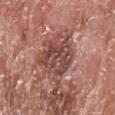- biopsy status — total-body-photography surveillance lesion; no biopsy
- body site — the back
- image — ~15 mm crop, total-body skin-cancer survey
- patient — male, in their mid- to late 60s